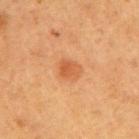No biopsy was performed on this lesion — it was imaged during a full skin examination and was not determined to be concerning. Cropped from a total-body skin-imaging series; the visible field is about 15 mm. The patient is a female aged 38 to 42. Automated tile analysis of the lesion measured a lesion area of about 5.5 mm² and an outline eccentricity of about 0.7 (0 = round, 1 = elongated). The analysis additionally found a border-irregularity rating of about 1.5/10, internal color variation of about 4 on a 0–10 scale, and peripheral color asymmetry of about 1.5. The tile uses cross-polarized illumination. The lesion is located on the right upper arm.